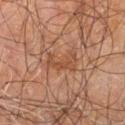This lesion was catalogued during total-body skin photography and was not selected for biopsy. A male patient aged 58 to 62. Located on the right leg. This image is a 15 mm lesion crop taken from a total-body photograph. The tile uses cross-polarized illumination. An algorithmic analysis of the crop reported a footprint of about 6 mm², a shape eccentricity near 0.9, and a shape-asymmetry score of about 0.45 (0 = symmetric). And it measured a classifier nevus-likeness of about 0/100 and lesion-presence confidence of about 90/100.